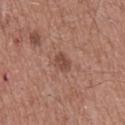workup — total-body-photography surveillance lesion; no biopsy | tile lighting — white-light illumination | lesion size — ~2.5 mm (longest diameter) | patient — male, about 50 years old | imaging modality — total-body-photography crop, ~15 mm field of view | body site — the mid back.